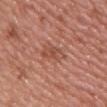Q: Was a biopsy performed?
A: total-body-photography surveillance lesion; no biopsy
Q: Patient demographics?
A: female, aged approximately 50
Q: What is the imaging modality?
A: 15 mm crop, total-body photography
Q: Lesion location?
A: the front of the torso